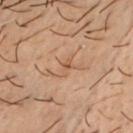Impression: Imaged during a routine full-body skin examination; the lesion was not biopsied and no histopathology is available. Background: The subject is a male aged around 50. Located on the chest. A 15 mm close-up tile from a total-body photography series done for melanoma screening. About 3 mm across. Captured under cross-polarized illumination.